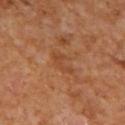workup: catalogued during a skin exam; not biopsied | patient: male, aged 63–67 | site: the mid back | TBP lesion metrics: a lesion color around L≈43 a*≈23 b*≈34 in CIELAB, a lesion–skin lightness drop of about 5, and a normalized border contrast of about 5 | size: ≈3.5 mm | image: ~15 mm tile from a whole-body skin photo | lighting: cross-polarized.